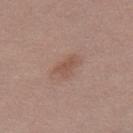Case summary:
- follow-up — catalogued during a skin exam; not biopsied
- subject — female, aged around 40
- image source — ~15 mm tile from a whole-body skin photo
- site — the right thigh